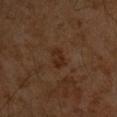The lesion was tiled from a total-body skin photograph and was not biopsied. The patient is a male roughly 60 years of age. This image is a 15 mm lesion crop taken from a total-body photograph. The recorded lesion diameter is about 2.5 mm. The lesion is located on the left upper arm.